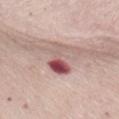Q: Is there a histopathology result?
A: imaged on a skin check; not biopsied
Q: What did automated image analysis measure?
A: roughly 14 lightness units darker than nearby skin and a normalized lesion–skin contrast near 9; border irregularity of about 4.5 on a 0–10 scale, a color-variation rating of about 10/10, and radial color variation of about 6.5; a classifier nevus-likeness of about 0/100 and a detector confidence of about 95 out of 100 that the crop contains a lesion
Q: Where on the body is the lesion?
A: the abdomen
Q: Who is the patient?
A: female, aged approximately 70
Q: How was this image acquired?
A: 15 mm crop, total-body photography
Q: How large is the lesion?
A: ~4 mm (longest diameter)